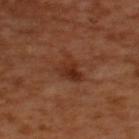A 15 mm crop from a total-body photograph taken for skin-cancer surveillance.
Located on the back.
The subject is a male in their 50s.